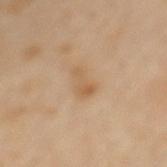{
  "site": "mid back",
  "patient": {
    "sex": "male",
    "age_approx": 65
  },
  "lesion_size": {
    "long_diameter_mm_approx": 3.0
  },
  "lighting": "cross-polarized",
  "image": {
    "source": "total-body photography crop",
    "field_of_view_mm": 15
  }
}